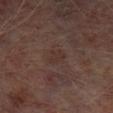workup=total-body-photography surveillance lesion; no biopsy